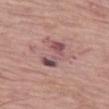Recorded during total-body skin imaging; not selected for excision or biopsy.
This image is a 15 mm lesion crop taken from a total-body photograph.
The recorded lesion diameter is about 4.5 mm.
A female patient approximately 85 years of age or older.
From the left thigh.